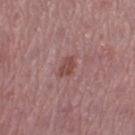- follow-up — total-body-photography surveillance lesion; no biopsy
- location — the leg
- image — total-body-photography crop, ~15 mm field of view
- image-analysis metrics — an area of roughly 4 mm² and a symmetry-axis asymmetry near 0.25; an average lesion color of about L≈47 a*≈23 b*≈22 (CIELAB), roughly 9 lightness units darker than nearby skin, and a lesion-to-skin contrast of about 7 (normalized; higher = more distinct); a within-lesion color-variation index near 2.5/10; a nevus-likeness score of about 15/100 and a detector confidence of about 100 out of 100 that the crop contains a lesion
- patient — female, aged approximately 45